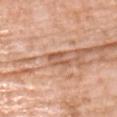workup: catalogued during a skin exam; not biopsied.